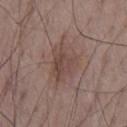Part of a total-body skin-imaging series; this lesion was reviewed on a skin check and was not flagged for biopsy. An algorithmic analysis of the crop reported an area of roughly 11 mm², a shape eccentricity near 0.55, and a symmetry-axis asymmetry near 0.35. The software also gave an average lesion color of about L≈45 a*≈17 b*≈22 (CIELAB), about 7 CIELAB-L* units darker than the surrounding skin, and a normalized lesion–skin contrast near 6. The analysis additionally found a classifier nevus-likeness of about 10/100 and a detector confidence of about 100 out of 100 that the crop contains a lesion. A region of skin cropped from a whole-body photographic capture, roughly 15 mm wide. The tile uses white-light illumination. The lesion is on the front of the torso. A male patient roughly 65 years of age.